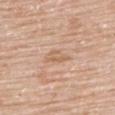notes=no biopsy performed (imaged during a skin exam) | image source=15 mm crop, total-body photography | subject=female, in their mid-80s or older | body site=the upper back | tile lighting=white-light illumination | lesion size=≈3 mm.